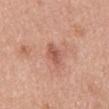workup: no biopsy performed (imaged during a skin exam)